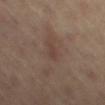Recorded during total-body skin imaging; not selected for excision or biopsy.